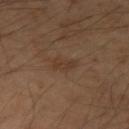Impression: The lesion was photographed on a routine skin check and not biopsied; there is no pathology result.